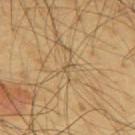Clinical impression: The lesion was tiled from a total-body skin photograph and was not biopsied. Acquisition and patient details: On the upper back. About 1.5 mm across. Captured under cross-polarized illumination. A 15 mm crop from a total-body photograph taken for skin-cancer surveillance. A male subject, in their mid- to late 60s.